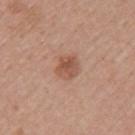Part of a total-body skin-imaging series; this lesion was reviewed on a skin check and was not flagged for biopsy.
The lesion is located on the left upper arm.
Imaged with white-light lighting.
Cropped from a whole-body photographic skin survey; the tile spans about 15 mm.
A female patient, about 55 years old.
Longest diameter approximately 2.5 mm.